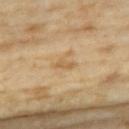Notes:
* workup · no biopsy performed (imaged during a skin exam)
* lesion size · ~2.5 mm (longest diameter)
* patient · female, aged 73 to 77
* body site · the chest
* lighting · cross-polarized illumination
* imaging modality · total-body-photography crop, ~15 mm field of view